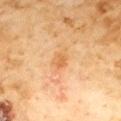workup: no biopsy performed (imaged during a skin exam) | subject: male, aged approximately 60 | illumination: cross-polarized | imaging modality: ~15 mm tile from a whole-body skin photo | anatomic site: the chest | lesion size: about 2.5 mm | automated metrics: roughly 7 lightness units darker than nearby skin and a lesion-to-skin contrast of about 6 (normalized; higher = more distinct); border irregularity of about 2 on a 0–10 scale, a within-lesion color-variation index near 2.5/10, and peripheral color asymmetry of about 1.